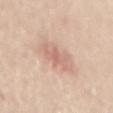| key | value |
|---|---|
| notes | no biopsy performed (imaged during a skin exam) |
| location | the lower back |
| acquisition | ~15 mm crop, total-body skin-cancer survey |
| automated lesion analysis | a footprint of about 7.5 mm², a shape eccentricity near 0.25, and two-axis asymmetry of about 0.3; a border-irregularity rating of about 5.5/10 and a within-lesion color-variation index near 2.5/10; a nevus-likeness score of about 5/100 and a detector confidence of about 100 out of 100 that the crop contains a lesion |
| diameter | ≈3.5 mm |
| patient | male, aged around 65 |